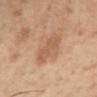{"biopsy_status": "not biopsied; imaged during a skin examination", "patient": {"sex": "male", "age_approx": 55}, "site": "left lower leg", "lighting": "cross-polarized", "image": {"source": "total-body photography crop", "field_of_view_mm": 15}}